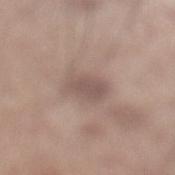notes = no biopsy performed (imaged during a skin exam); subject = male, roughly 60 years of age; anatomic site = the left lower leg; image = ~15 mm tile from a whole-body skin photo.